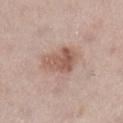illumination = white-light | body site = the right lower leg | patient = female, aged approximately 40 | lesion diameter = about 5 mm | imaging modality = total-body-photography crop, ~15 mm field of view.